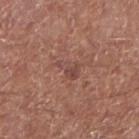Case summary:
• follow-up — total-body-photography surveillance lesion; no biopsy
• diameter — ≈3 mm
• illumination — white-light illumination
• acquisition — 15 mm crop, total-body photography
• automated lesion analysis — a border-irregularity rating of about 6.5/10, a within-lesion color-variation index near 1.5/10, and radial color variation of about 0.5
• subject — male, aged around 65
• site — the right forearm